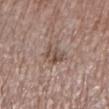Impression:
Recorded during total-body skin imaging; not selected for excision or biopsy.
Acquisition and patient details:
Located on the left lower leg. The patient is a male aged 63 to 67. Automated image analysis of the tile measured an area of roughly 5.5 mm², an outline eccentricity of about 0.7 (0 = round, 1 = elongated), and a shape-asymmetry score of about 0.25 (0 = symmetric). The analysis additionally found an average lesion color of about L≈49 a*≈16 b*≈23 (CIELAB). The software also gave an automated nevus-likeness rating near 0 out of 100 and a lesion-detection confidence of about 100/100. Imaged with white-light lighting. A 15 mm crop from a total-body photograph taken for skin-cancer surveillance. The recorded lesion diameter is about 3.5 mm.